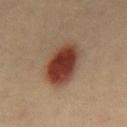The lesion was tiled from a total-body skin photograph and was not biopsied. The lesion is located on the mid back. Approximately 6 mm at its widest. Automated image analysis of the tile measured border irregularity of about 1.5 on a 0–10 scale, internal color variation of about 6.5 on a 0–10 scale, and radial color variation of about 2. It also reported an automated nevus-likeness rating near 100 out of 100 and a detector confidence of about 100 out of 100 that the crop contains a lesion. A male patient roughly 40 years of age. Imaged with cross-polarized lighting. This image is a 15 mm lesion crop taken from a total-body photograph.